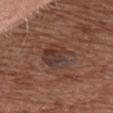<lesion>
<lesion_size>
  <long_diameter_mm_approx>4.0</long_diameter_mm_approx>
</lesion_size>
<image>
  <source>total-body photography crop</source>
  <field_of_view_mm>15</field_of_view_mm>
</image>
<lighting>white-light</lighting>
<patient>
  <sex>female</sex>
  <age_approx>75</age_approx>
</patient>
<site>front of the torso</site>
</lesion>